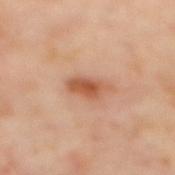Clinical impression: The lesion was tiled from a total-body skin photograph and was not biopsied. Image and clinical context: The total-body-photography lesion software estimated an average lesion color of about L≈55 a*≈25 b*≈34 (CIELAB), a lesion–skin lightness drop of about 12, and a normalized lesion–skin contrast near 8. And it measured a color-variation rating of about 3.5/10. The analysis additionally found an automated nevus-likeness rating near 90 out of 100 and a detector confidence of about 100 out of 100 that the crop contains a lesion. Captured under cross-polarized illumination. This image is a 15 mm lesion crop taken from a total-body photograph. The lesion is located on the upper back. Approximately 4 mm at its widest. A female patient roughly 60 years of age.